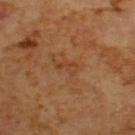Captured during whole-body skin photography for melanoma surveillance; the lesion was not biopsied.